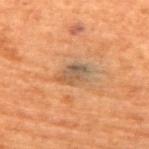| key | value |
|---|---|
| workup | imaged on a skin check; not biopsied |
| subject | female, aged approximately 80 |
| location | the chest |
| tile lighting | cross-polarized illumination |
| acquisition | 15 mm crop, total-body photography |